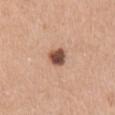Impression: Imaged during a routine full-body skin examination; the lesion was not biopsied and no histopathology is available. Acquisition and patient details: Measured at roughly 2.5 mm in maximum diameter. This is a white-light tile. An algorithmic analysis of the crop reported border irregularity of about 1.5 on a 0–10 scale, a color-variation rating of about 5.5/10, and radial color variation of about 2. The analysis additionally found a nevus-likeness score of about 95/100. Located on the arm. A female subject, aged around 45. Cropped from a whole-body photographic skin survey; the tile spans about 15 mm.